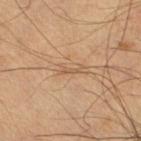No biopsy was performed on this lesion — it was imaged during a full skin examination and was not determined to be concerning. Automated tile analysis of the lesion measured an area of roughly 2 mm², a shape eccentricity near 0.95, and a symmetry-axis asymmetry near 0.55. The analysis additionally found border irregularity of about 6 on a 0–10 scale, internal color variation of about 0 on a 0–10 scale, and radial color variation of about 0. The lesion's longest dimension is about 3 mm. Imaged with cross-polarized lighting. A male patient, approximately 40 years of age. From the right lower leg. A 15 mm crop from a total-body photograph taken for skin-cancer surveillance.